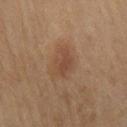{
  "image": {
    "source": "total-body photography crop",
    "field_of_view_mm": 15
  },
  "lesion_size": {
    "long_diameter_mm_approx": 3.5
  },
  "site": "right thigh",
  "lighting": "cross-polarized",
  "patient": {
    "sex": "female",
    "age_approx": 60
  }
}